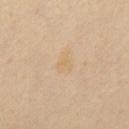follow-up=imaged on a skin check; not biopsied | imaging modality=total-body-photography crop, ~15 mm field of view | patient=male, in their mid- to late 50s | lighting=cross-polarized illumination | TBP lesion metrics=a lesion color around L≈69 a*≈13 b*≈38 in CIELAB, roughly 4 lightness units darker than nearby skin, and a normalized lesion–skin contrast near 4.5; an automated nevus-likeness rating near 0 out of 100 | size=≈3 mm | location=the abdomen.